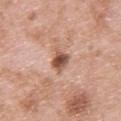workup: total-body-photography surveillance lesion; no biopsy | patient: male, about 50 years old | body site: the chest | imaging modality: ~15 mm crop, total-body skin-cancer survey | size: ~2.5 mm (longest diameter) | automated metrics: an area of roughly 4.5 mm²; a lesion color around L≈53 a*≈22 b*≈29 in CIELAB and a lesion-to-skin contrast of about 10 (normalized; higher = more distinct); a classifier nevus-likeness of about 90/100 and lesion-presence confidence of about 100/100.